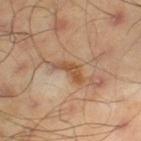Imaged during a routine full-body skin examination; the lesion was not biopsied and no histopathology is available. The lesion-visualizer software estimated an area of roughly 5 mm², an outline eccentricity of about 0.9 (0 = round, 1 = elongated), and two-axis asymmetry of about 0.5. The software also gave a mean CIELAB color near L≈52 a*≈20 b*≈34, a lesion–skin lightness drop of about 10, and a lesion-to-skin contrast of about 7.5 (normalized; higher = more distinct). It also reported border irregularity of about 5.5 on a 0–10 scale, a color-variation rating of about 2/10, and peripheral color asymmetry of about 0.5. It also reported a nevus-likeness score of about 0/100. Longest diameter approximately 4 mm. A region of skin cropped from a whole-body photographic capture, roughly 15 mm wide. The lesion is located on the left thigh. Imaged with cross-polarized lighting. A male subject, in their mid- to late 40s.